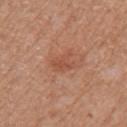body site — the right upper arm | tile lighting — white-light illumination | TBP lesion metrics — an area of roughly 5 mm², an outline eccentricity of about 0.65 (0 = round, 1 = elongated), and two-axis asymmetry of about 0.25; a border-irregularity rating of about 3/10 and radial color variation of about 0.5 | size — ≈3 mm | patient — female, in their mid-70s | acquisition — ~15 mm tile from a whole-body skin photo.